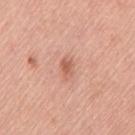This lesion was catalogued during total-body skin photography and was not selected for biopsy. On the leg. A female patient aged around 55. A 15 mm close-up extracted from a 3D total-body photography capture.